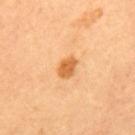A female patient about 50 years old.
Captured under cross-polarized illumination.
A region of skin cropped from a whole-body photographic capture, roughly 15 mm wide.